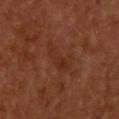<record>
  <biopsy_status>not biopsied; imaged during a skin examination</biopsy_status>
  <lighting>cross-polarized</lighting>
  <site>upper back</site>
  <image>
    <source>total-body photography crop</source>
    <field_of_view_mm>15</field_of_view_mm>
  </image>
  <patient>
    <sex>male</sex>
    <age_approx>60</age_approx>
  </patient>
</record>